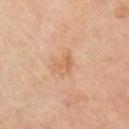<record>
<biopsy_status>not biopsied; imaged during a skin examination</biopsy_status>
<site>right upper arm</site>
<automated_metrics>
  <nevus_likeness_0_100>0</nevus_likeness_0_100>
  <lesion_detection_confidence_0_100>100</lesion_detection_confidence_0_100>
</automated_metrics>
<patient>
  <sex>male</sex>
  <age_approx>65</age_approx>
</patient>
<lesion_size>
  <long_diameter_mm_approx>2.5</long_diameter_mm_approx>
</lesion_size>
<image>
  <source>total-body photography crop</source>
  <field_of_view_mm>15</field_of_view_mm>
</image>
<lighting>cross-polarized</lighting>
</record>